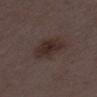Clinical impression:
This lesion was catalogued during total-body skin photography and was not selected for biopsy.
Image and clinical context:
The patient is a female in their 30s. From the leg. Automated image analysis of the tile measured a mean CIELAB color near L≈28 a*≈14 b*≈17, a lesion–skin lightness drop of about 7, and a normalized border contrast of about 8. The software also gave a border-irregularity rating of about 2.5/10 and internal color variation of about 3 on a 0–10 scale. The analysis additionally found an automated nevus-likeness rating near 55 out of 100. A 15 mm close-up extracted from a 3D total-body photography capture. This is a white-light tile.